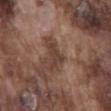This lesion was catalogued during total-body skin photography and was not selected for biopsy.